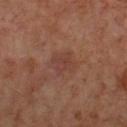The lesion was photographed on a routine skin check and not biopsied; there is no pathology result.
The lesion's longest dimension is about 3 mm.
Cropped from a total-body skin-imaging series; the visible field is about 15 mm.
The subject is a male aged 63–67.
Automated image analysis of the tile measured a lesion color around L≈39 a*≈21 b*≈25 in CIELAB, roughly 5 lightness units darker than nearby skin, and a normalized border contrast of about 5. The analysis additionally found border irregularity of about 2.5 on a 0–10 scale, internal color variation of about 2.5 on a 0–10 scale, and radial color variation of about 1. The software also gave an automated nevus-likeness rating near 0 out of 100.